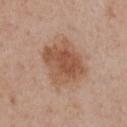| feature | finding |
|---|---|
| notes | no biopsy performed (imaged during a skin exam) |
| subject | female, approximately 55 years of age |
| tile lighting | white-light |
| location | the chest |
| size | about 5.5 mm |
| image | 15 mm crop, total-body photography |